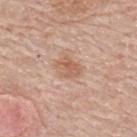Recorded during total-body skin imaging; not selected for excision or biopsy. The recorded lesion diameter is about 3 mm. A male patient, aged 68 to 72. The lesion is located on the upper back. This image is a 15 mm lesion crop taken from a total-body photograph.